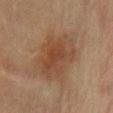Captured during whole-body skin photography for melanoma surveillance; the lesion was not biopsied.
The lesion is located on the abdomen.
The total-body-photography lesion software estimated a mean CIELAB color near L≈38 a*≈17 b*≈27. And it measured a classifier nevus-likeness of about 40/100 and a lesion-detection confidence of about 100/100.
A female subject, in their 80s.
The recorded lesion diameter is about 6.5 mm.
A roughly 15 mm field-of-view crop from a total-body skin photograph.
This is a cross-polarized tile.